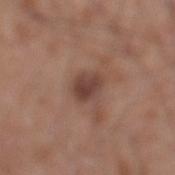follow-up=catalogued during a skin exam; not biopsied | diameter=≈3.5 mm | patient=male, aged 18 to 22 | body site=the leg | imaging modality=total-body-photography crop, ~15 mm field of view | illumination=white-light.